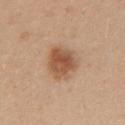workup = total-body-photography surveillance lesion; no biopsy
subject = female, aged approximately 25
imaging modality = ~15 mm tile from a whole-body skin photo
location = the upper back